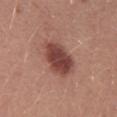Impression: This lesion was catalogued during total-body skin photography and was not selected for biopsy. Background: Automated image analysis of the tile measured a lesion area of about 15 mm², an eccentricity of roughly 0.75, and a shape-asymmetry score of about 0.15 (0 = symmetric). It also reported a mean CIELAB color near L≈45 a*≈24 b*≈24, roughly 13 lightness units darker than nearby skin, and a lesion-to-skin contrast of about 10 (normalized; higher = more distinct). This image is a 15 mm lesion crop taken from a total-body photograph. A female subject in their mid-20s. About 5.5 mm across. The lesion is located on the abdomen.